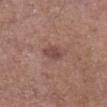| key | value |
|---|---|
| workup | catalogued during a skin exam; not biopsied |
| body site | the leg |
| patient | male, roughly 60 years of age |
| acquisition | ~15 mm tile from a whole-body skin photo |
| diameter | ≈2.5 mm |
| lighting | white-light illumination |